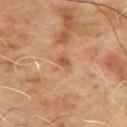A male patient roughly 65 years of age.
The lesion's longest dimension is about 2.5 mm.
A lesion tile, about 15 mm wide, cut from a 3D total-body photograph.
On the upper back.
This is a cross-polarized tile.
An algorithmic analysis of the crop reported a border-irregularity rating of about 3.5/10 and a color-variation rating of about 4/10.